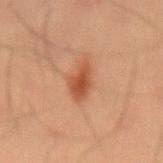notes — catalogued during a skin exam; not biopsied | TBP lesion metrics — a footprint of about 6.5 mm², an outline eccentricity of about 0.85 (0 = round, 1 = elongated), and a symmetry-axis asymmetry near 0.3; an average lesion color of about L≈44 a*≈24 b*≈32 (CIELAB), about 10 CIELAB-L* units darker than the surrounding skin, and a lesion-to-skin contrast of about 8 (normalized; higher = more distinct) | patient — male, roughly 55 years of age | illumination — cross-polarized illumination | site — the front of the torso | acquisition — ~15 mm crop, total-body skin-cancer survey.